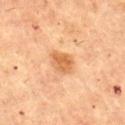Findings:
– workup · catalogued during a skin exam; not biopsied
– body site · the mid back
– lighting · cross-polarized
– automated lesion analysis · an area of roughly 5.5 mm², an outline eccentricity of about 0.55 (0 = round, 1 = elongated), and a symmetry-axis asymmetry near 0.2; lesion-presence confidence of about 100/100
– image source · ~15 mm tile from a whole-body skin photo
– lesion size · ~3 mm (longest diameter)
– subject · male, aged approximately 65